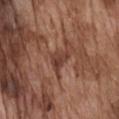Q: Is there a histopathology result?
A: no biopsy performed (imaged during a skin exam)
Q: What did automated image analysis measure?
A: a footprint of about 4 mm² and a symmetry-axis asymmetry near 0.45; a border-irregularity index near 4/10 and a peripheral color-asymmetry measure near 1; a classifier nevus-likeness of about 0/100 and a lesion-detection confidence of about 65/100
Q: How was the tile lit?
A: white-light illumination
Q: What kind of image is this?
A: total-body-photography crop, ~15 mm field of view
Q: Lesion location?
A: the front of the torso
Q: Who is the patient?
A: male, in their mid- to late 70s